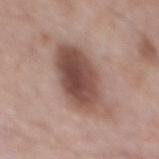The lesion was tiled from a total-body skin photograph and was not biopsied. A male patient, aged 53–57. The lesion is located on the back. The lesion-visualizer software estimated border irregularity of about 2.5 on a 0–10 scale, a color-variation rating of about 7/10, and radial color variation of about 2.5. It also reported an automated nevus-likeness rating near 95 out of 100 and a lesion-detection confidence of about 100/100. Imaged with white-light lighting. A region of skin cropped from a whole-body photographic capture, roughly 15 mm wide.